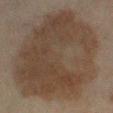{
  "biopsy_status": "not biopsied; imaged during a skin examination",
  "site": "left lower leg",
  "patient": {
    "sex": "male",
    "age_approx": 65
  },
  "automated_metrics": {
    "shape_asymmetry": 0.15,
    "nevus_likeness_0_100": 10,
    "lesion_detection_confidence_0_100": 95
  },
  "image": {
    "source": "total-body photography crop",
    "field_of_view_mm": 15
  },
  "lesion_size": {
    "long_diameter_mm_approx": 12.0
  }
}